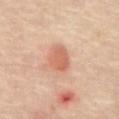<lesion>
<lighting>cross-polarized</lighting>
<image>
  <source>total-body photography crop</source>
  <field_of_view_mm>15</field_of_view_mm>
</image>
<site>abdomen</site>
<automated_metrics>
  <cielab_L>64</cielab_L>
  <cielab_a>26</cielab_a>
  <cielab_b>33</cielab_b>
  <vs_skin_darker_L>10.0</vs_skin_darker_L>
  <vs_skin_contrast_norm>6.5</vs_skin_contrast_norm>
  <nevus_likeness_0_100>95</nevus_likeness_0_100>
  <lesion_detection_confidence_0_100>100</lesion_detection_confidence_0_100>
</automated_metrics>
</lesion>